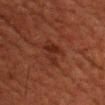Q: Is there a histopathology result?
A: total-body-photography surveillance lesion; no biopsy
Q: What lighting was used for the tile?
A: cross-polarized illumination
Q: What is the anatomic site?
A: the chest
Q: Automated lesion metrics?
A: a lesion area of about 6.5 mm², a shape eccentricity near 0.75, and a symmetry-axis asymmetry near 0.35
Q: What kind of image is this?
A: total-body-photography crop, ~15 mm field of view
Q: How large is the lesion?
A: about 4 mm
Q: Who is the patient?
A: male, aged 48 to 52